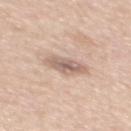diameter: ≈4 mm
acquisition: 15 mm crop, total-body photography
subject: male, aged 48 to 52
lighting: white-light
TBP lesion metrics: a mean CIELAB color near L≈62 a*≈17 b*≈25, roughly 11 lightness units darker than nearby skin, and a lesion-to-skin contrast of about 7 (normalized; higher = more distinct)
body site: the mid back